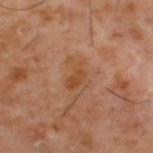{"biopsy_status": "not biopsied; imaged during a skin examination", "automated_metrics": {"eccentricity": 0.7, "shape_asymmetry": 0.5, "cielab_L": 44, "cielab_a": 21, "cielab_b": 33, "vs_skin_darker_L": 6.0, "vs_skin_contrast_norm": 6.5}, "patient": {"sex": "male", "age_approx": 60}, "lesion_size": {"long_diameter_mm_approx": 3.5}, "image": {"source": "total-body photography crop", "field_of_view_mm": 15}, "site": "upper back"}